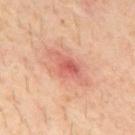| feature | finding |
|---|---|
| workup | no biopsy performed (imaged during a skin exam) |
| site | the mid back |
| image source | 15 mm crop, total-body photography |
| lesion size | about 6 mm |
| patient | male, roughly 30 years of age |
| illumination | cross-polarized illumination |